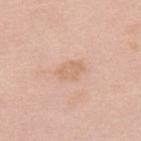workup: catalogued during a skin exam; not biopsied
location: the upper back
image: ~15 mm tile from a whole-body skin photo
patient: female, roughly 65 years of age
tile lighting: white-light illumination
image-analysis metrics: an area of roughly 5 mm², an outline eccentricity of about 0.8 (0 = round, 1 = elongated), and two-axis asymmetry of about 0.25; an average lesion color of about L≈67 a*≈19 b*≈32 (CIELAB), a lesion–skin lightness drop of about 7, and a normalized lesion–skin contrast near 5; a border-irregularity rating of about 2.5/10, a color-variation rating of about 2/10, and radial color variation of about 0.5